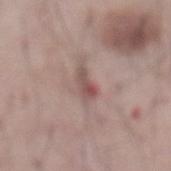This lesion was catalogued during total-body skin photography and was not selected for biopsy.
From the abdomen.
The subject is a male roughly 55 years of age.
A lesion tile, about 15 mm wide, cut from a 3D total-body photograph.